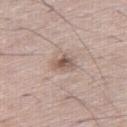biopsy_status: not biopsied; imaged during a skin examination
image:
  source: total-body photography crop
  field_of_view_mm: 15
patient:
  sex: male
  age_approx: 70
lesion_size:
  long_diameter_mm_approx: 2.5
automated_metrics:
  cielab_L: 55
  cielab_a: 16
  cielab_b: 24
  vs_skin_darker_L: 12.0
site: right thigh
lighting: white-light